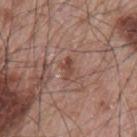Recorded during total-body skin imaging; not selected for excision or biopsy.
Cropped from a total-body skin-imaging series; the visible field is about 15 mm.
Measured at roughly 2.5 mm in maximum diameter.
This is a white-light tile.
Located on the arm.
A male subject, in their mid- to late 60s.